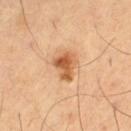This lesion was catalogued during total-body skin photography and was not selected for biopsy. A male subject, aged approximately 65. The recorded lesion diameter is about 3.5 mm. From the left thigh. A lesion tile, about 15 mm wide, cut from a 3D total-body photograph. Imaged with cross-polarized lighting.